Located on the head or neck. This image is a 15 mm lesion crop taken from a total-body photograph. The patient is a male aged around 60: 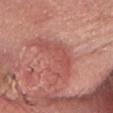Conclusion:
On biopsy, histopathology showed a squamous cell carcinoma in situ — a skin cancer.